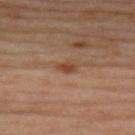The lesion was photographed on a routine skin check and not biopsied; there is no pathology result.